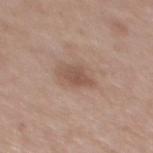Notes:
* workup — no biopsy performed (imaged during a skin exam)
* TBP lesion metrics — border irregularity of about 2.5 on a 0–10 scale, a within-lesion color-variation index near 2/10, and peripheral color asymmetry of about 0.5; a lesion-detection confidence of about 100/100
* subject — female, about 40 years old
* image — 15 mm crop, total-body photography
* lesion size — about 3.5 mm
* body site — the mid back